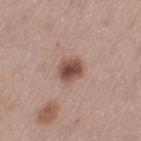Assessment:
This lesion was catalogued during total-body skin photography and was not selected for biopsy.
Background:
This image is a 15 mm lesion crop taken from a total-body photograph. An algorithmic analysis of the crop reported a mean CIELAB color near L≈48 a*≈20 b*≈25, a lesion–skin lightness drop of about 14, and a normalized border contrast of about 10. The software also gave internal color variation of about 4 on a 0–10 scale and peripheral color asymmetry of about 1. The analysis additionally found a classifier nevus-likeness of about 95/100. From the left thigh. The patient is a female in their mid-60s.